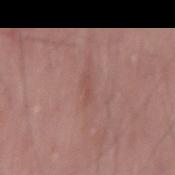A male subject, aged around 50. An algorithmic analysis of the crop reported a footprint of about 2.5 mm², an eccentricity of roughly 0.95, and a shape-asymmetry score of about 0.3 (0 = symmetric). It also reported a border-irregularity index near 4/10, a color-variation rating of about 0/10, and radial color variation of about 0. The software also gave a classifier nevus-likeness of about 0/100 and lesion-presence confidence of about 85/100. Approximately 3 mm at its widest. A 15 mm crop from a total-body photograph taken for skin-cancer surveillance. Located on the left thigh. The tile uses white-light illumination.